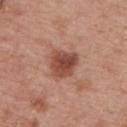Case summary:
* patient: male, in their mid-60s
* lesion diameter: ≈3.5 mm
* anatomic site: the upper back
* tile lighting: white-light illumination
* imaging modality: ~15 mm tile from a whole-body skin photo